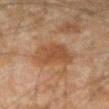Clinical impression: Part of a total-body skin-imaging series; this lesion was reviewed on a skin check and was not flagged for biopsy. Acquisition and patient details: The recorded lesion diameter is about 4.5 mm. An algorithmic analysis of the crop reported a footprint of about 12 mm² and two-axis asymmetry of about 0.3. The software also gave a border-irregularity index near 3/10, a color-variation rating of about 2/10, and a peripheral color-asymmetry measure near 0.5. The lesion is on the arm. A roughly 15 mm field-of-view crop from a total-body skin photograph. A male patient aged around 45.